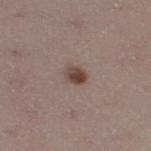No biopsy was performed on this lesion — it was imaged during a full skin examination and was not determined to be concerning.
The lesion is located on the right thigh.
A female subject about 25 years old.
The lesion's longest dimension is about 2.5 mm.
Imaged with white-light lighting.
A 15 mm close-up tile from a total-body photography series done for melanoma screening.
The lesion-visualizer software estimated a lesion area of about 4 mm², an outline eccentricity of about 0.65 (0 = round, 1 = elongated), and a symmetry-axis asymmetry near 0.25. And it measured a mean CIELAB color near L≈44 a*≈17 b*≈22 and a lesion-to-skin contrast of about 9.5 (normalized; higher = more distinct). The analysis additionally found a border-irregularity index near 2/10, a within-lesion color-variation index near 5.5/10, and a peripheral color-asymmetry measure near 2. The analysis additionally found a classifier nevus-likeness of about 100/100 and a detector confidence of about 100 out of 100 that the crop contains a lesion.